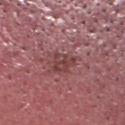Assessment: No biopsy was performed on this lesion — it was imaged during a full skin examination and was not determined to be concerning. Background: The total-body-photography lesion software estimated a shape eccentricity near 0.3 and two-axis asymmetry of about 0.35. It also reported a mean CIELAB color near L≈42 a*≈25 b*≈20, about 8 CIELAB-L* units darker than the surrounding skin, and a normalized border contrast of about 6.5. The subject is a male aged approximately 40. The lesion is on the head or neck. Longest diameter approximately 3 mm. A close-up tile cropped from a whole-body skin photograph, about 15 mm across.